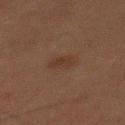Findings:
* follow-up · imaged on a skin check; not biopsied
* diameter · ~3 mm (longest diameter)
* image-analysis metrics · two-axis asymmetry of about 0.3; a lesion color around L≈27 a*≈14 b*≈22 in CIELAB, about 4 CIELAB-L* units darker than the surrounding skin, and a normalized lesion–skin contrast near 5.5; border irregularity of about 2.5 on a 0–10 scale, internal color variation of about 2 on a 0–10 scale, and radial color variation of about 1; a nevus-likeness score of about 65/100
* illumination · cross-polarized
* site · the mid back
* subject · male, about 50 years old
* imaging modality · 15 mm crop, total-body photography